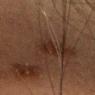Findings:
- notes — no biopsy performed (imaged during a skin exam)
- diameter — about 2.5 mm
- image source — total-body-photography crop, ~15 mm field of view
- lighting — cross-polarized
- image-analysis metrics — an area of roughly 4.5 mm², a shape eccentricity near 0.5, and two-axis asymmetry of about 0.25; a lesion color around L≈20 a*≈15 b*≈20 in CIELAB, a lesion–skin lightness drop of about 6, and a normalized lesion–skin contrast near 7
- location — the head or neck
- patient — female, roughly 55 years of age